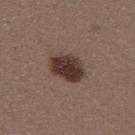Part of a total-body skin-imaging series; this lesion was reviewed on a skin check and was not flagged for biopsy.
Measured at roughly 4.5 mm in maximum diameter.
A 15 mm crop from a total-body photograph taken for skin-cancer surveillance.
Imaged with white-light lighting.
The lesion is located on the left thigh.
A female subject aged around 30.